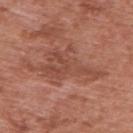<lesion>
<lesion_size>
  <long_diameter_mm_approx>7.0</long_diameter_mm_approx>
</lesion_size>
<site>upper back</site>
<automated_metrics>
  <cielab_L>48</cielab_L>
  <cielab_a>24</cielab_a>
  <cielab_b>28</cielab_b>
  <vs_skin_darker_L>7.0</vs_skin_darker_L>
  <vs_skin_contrast_norm>5.5</vs_skin_contrast_norm>
</automated_metrics>
<image>
  <source>total-body photography crop</source>
  <field_of_view_mm>15</field_of_view_mm>
</image>
<patient>
  <sex>male</sex>
  <age_approx>70</age_approx>
</patient>
</lesion>